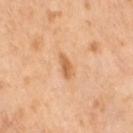Imaged during a routine full-body skin examination; the lesion was not biopsied and no histopathology is available. The lesion is on the right thigh. A female subject, aged approximately 55. Automated tile analysis of the lesion measured an area of roughly 4 mm² and an eccentricity of roughly 0.85. The software also gave a mean CIELAB color near L≈65 a*≈24 b*≈41 and roughly 10 lightness units darker than nearby skin. And it measured internal color variation of about 2 on a 0–10 scale and radial color variation of about 0.5. The lesion's longest dimension is about 3 mm. Captured under cross-polarized illumination. A lesion tile, about 15 mm wide, cut from a 3D total-body photograph.